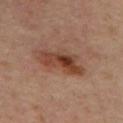Impression:
Recorded during total-body skin imaging; not selected for excision or biopsy.
Acquisition and patient details:
From the mid back. A female patient about 45 years old. This is a cross-polarized tile. The total-body-photography lesion software estimated a mean CIELAB color near L≈40 a*≈21 b*≈28. And it measured a border-irregularity index near 4/10 and radial color variation of about 2.5. The analysis additionally found a classifier nevus-likeness of about 80/100 and a lesion-detection confidence of about 100/100. The lesion's longest dimension is about 6 mm. Cropped from a whole-body photographic skin survey; the tile spans about 15 mm.